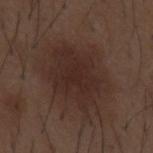Context: Cropped from a whole-body photographic skin survey; the tile spans about 15 mm. From the mid back. The patient is a male approximately 50 years of age. The recorded lesion diameter is about 8 mm. The tile uses white-light illumination.